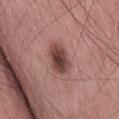A male patient, aged 53 to 57. A close-up tile cropped from a whole-body skin photograph, about 15 mm across.